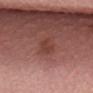Recorded during total-body skin imaging; not selected for excision or biopsy.
Automated image analysis of the tile measured two-axis asymmetry of about 0.35. The software also gave a lesion-detection confidence of about 100/100.
The lesion is located on the back.
Measured at roughly 3.5 mm in maximum diameter.
A male subject, about 40 years old.
A region of skin cropped from a whole-body photographic capture, roughly 15 mm wide.
The tile uses white-light illumination.